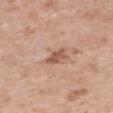Case summary:
* notes: imaged on a skin check; not biopsied
* subject: female, aged 38–42
* anatomic site: the chest
* diameter: ≈3 mm
* acquisition: ~15 mm tile from a whole-body skin photo
* lighting: white-light illumination